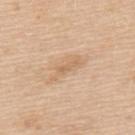The lesion-visualizer software estimated a footprint of about 2.5 mm² and a shape-asymmetry score of about 0.4 (0 = symmetric). And it measured a color-variation rating of about 0/10 and a peripheral color-asymmetry measure near 0. The analysis additionally found a nevus-likeness score of about 0/100 and lesion-presence confidence of about 95/100. Measured at roughly 3 mm in maximum diameter. A region of skin cropped from a whole-body photographic capture, roughly 15 mm wide. A female patient, aged 53–57. The lesion is located on the upper back. Imaged with white-light lighting.